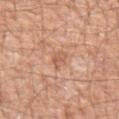workup: no biopsy performed (imaged during a skin exam)
lesion diameter: ≈2.5 mm
imaging modality: ~15 mm crop, total-body skin-cancer survey
site: the left forearm
subject: male, aged 48 to 52
tile lighting: white-light illumination
automated metrics: an area of roughly 3 mm² and a shape eccentricity near 0.8